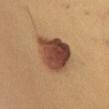Recorded during total-body skin imaging; not selected for excision or biopsy. An algorithmic analysis of the crop reported a footprint of about 19 mm² and a shape-asymmetry score of about 0.2 (0 = symmetric). It also reported a border-irregularity rating of about 2.5/10, internal color variation of about 8 on a 0–10 scale, and peripheral color asymmetry of about 3. From the arm. About 5 mm across. A female patient, aged 38–42. A 15 mm crop from a total-body photograph taken for skin-cancer surveillance.